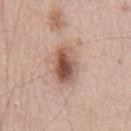Q: Was this lesion biopsied?
A: imaged on a skin check; not biopsied
Q: What lighting was used for the tile?
A: white-light illumination
Q: What is the imaging modality?
A: total-body-photography crop, ~15 mm field of view
Q: Automated lesion metrics?
A: a nevus-likeness score of about 95/100 and lesion-presence confidence of about 100/100
Q: Who is the patient?
A: male, approximately 55 years of age
Q: What is the anatomic site?
A: the chest